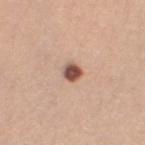{"biopsy_status": "not biopsied; imaged during a skin examination", "patient": {"sex": "female", "age_approx": 60}, "site": "left thigh", "image": {"source": "total-body photography crop", "field_of_view_mm": 15}}